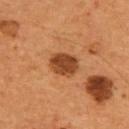Recorded during total-body skin imaging; not selected for excision or biopsy. This is a cross-polarized tile. A male subject aged approximately 55. The lesion is located on the upper back. A 15 mm close-up extracted from a 3D total-body photography capture. The recorded lesion diameter is about 4 mm.